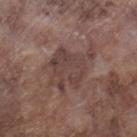A 15 mm close-up extracted from a 3D total-body photography capture.
The lesion is on the right lower leg.
The subject is a male aged around 75.